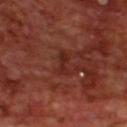The lesion was photographed on a routine skin check and not biopsied; there is no pathology result.
The lesion-visualizer software estimated an area of roughly 3.5 mm² and two-axis asymmetry of about 0.3. The analysis additionally found an average lesion color of about L≈26 a*≈26 b*≈25 (CIELAB), a lesion–skin lightness drop of about 6, and a normalized lesion–skin contrast near 6.5. It also reported an automated nevus-likeness rating near 0 out of 100 and lesion-presence confidence of about 90/100.
The lesion's longest dimension is about 3 mm.
A male subject aged 68 to 72.
A lesion tile, about 15 mm wide, cut from a 3D total-body photograph.
The lesion is located on the upper back.